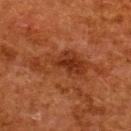Part of a total-body skin-imaging series; this lesion was reviewed on a skin check and was not flagged for biopsy. The tile uses cross-polarized illumination. An algorithmic analysis of the crop reported a within-lesion color-variation index near 4/10 and radial color variation of about 1. A 15 mm close-up tile from a total-body photography series done for melanoma screening. Longest diameter approximately 8.5 mm. The lesion is located on the upper back. The patient is a female aged 48–52.